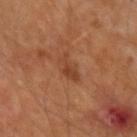Case summary:
* patient: male, aged around 65
* image source: ~15 mm crop, total-body skin-cancer survey
* illumination: cross-polarized illumination
* size: ~3 mm (longest diameter)
* site: the right upper arm
* image-analysis metrics: a within-lesion color-variation index near 2.5/10 and radial color variation of about 1; an automated nevus-likeness rating near 5 out of 100